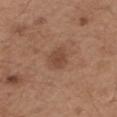| field | value |
|---|---|
| site | the left forearm |
| image source | total-body-photography crop, ~15 mm field of view |
| patient | male, aged 68–72 |
| lesion diameter | about 3 mm |